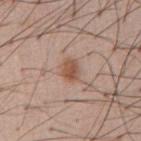workup: total-body-photography surveillance lesion; no biopsy
patient: male, aged 53–57
image: total-body-photography crop, ~15 mm field of view
tile lighting: white-light illumination
site: the abdomen